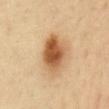Impression:
Recorded during total-body skin imaging; not selected for excision or biopsy.
Acquisition and patient details:
A female patient, aged 68–72. Automated tile analysis of the lesion measured a footprint of about 15 mm², an eccentricity of roughly 0.75, and two-axis asymmetry of about 0.2. The software also gave border irregularity of about 2 on a 0–10 scale, a color-variation rating of about 8.5/10, and a peripheral color-asymmetry measure near 2.5. A 15 mm close-up tile from a total-body photography series done for melanoma screening. The lesion is located on the abdomen. This is a cross-polarized tile. About 5.5 mm across.